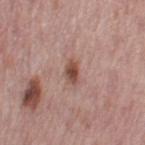Clinical impression: No biopsy was performed on this lesion — it was imaged during a full skin examination and was not determined to be concerning. Image and clinical context: Measured at roughly 3 mm in maximum diameter. A lesion tile, about 15 mm wide, cut from a 3D total-body photograph. The subject is a female roughly 50 years of age. Captured under white-light illumination. The lesion is on the left thigh. The lesion-visualizer software estimated an eccentricity of roughly 0.85 and a shape-asymmetry score of about 0.2 (0 = symmetric). It also reported a nevus-likeness score of about 95/100 and lesion-presence confidence of about 100/100.